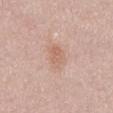patient:
  sex: female
  age_approx: 60
image:
  source: total-body photography crop
  field_of_view_mm: 15
lighting: white-light
site: mid back